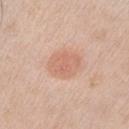biopsy_status: not biopsied; imaged during a skin examination
automated_metrics:
  border_irregularity_0_10: 2.0
  color_variation_0_10: 2.0
  peripheral_color_asymmetry: 0.5
lesion_size:
  long_diameter_mm_approx: 4.0
site: left upper arm
patient:
  sex: male
  age_approx: 55
image:
  source: total-body photography crop
  field_of_view_mm: 15